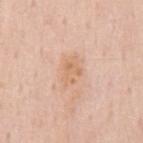follow-up: imaged on a skin check; not biopsied
anatomic site: the mid back
acquisition: 15 mm crop, total-body photography
TBP lesion metrics: border irregularity of about 4.5 on a 0–10 scale; a nevus-likeness score of about 0/100 and a lesion-detection confidence of about 100/100
diameter: ~3.5 mm (longest diameter)
subject: male, about 60 years old
illumination: white-light illumination